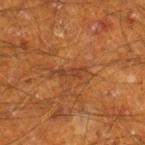Recorded during total-body skin imaging; not selected for excision or biopsy.
From the right lower leg.
The lesion's longest dimension is about 3 mm.
A male patient aged 58 to 62.
Captured under cross-polarized illumination.
A region of skin cropped from a whole-body photographic capture, roughly 15 mm wide.
Automated tile analysis of the lesion measured a border-irregularity index near 5.5/10, a within-lesion color-variation index near 0/10, and a peripheral color-asymmetry measure near 0. It also reported a nevus-likeness score of about 0/100 and a detector confidence of about 75 out of 100 that the crop contains a lesion.